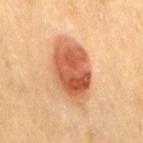workup: total-body-photography surveillance lesion; no biopsy
anatomic site: the right thigh
acquisition: total-body-photography crop, ~15 mm field of view
subject: female, aged approximately 40
automated metrics: an area of roughly 24 mm² and a symmetry-axis asymmetry near 0.1; a lesion–skin lightness drop of about 14 and a lesion-to-skin contrast of about 9.5 (normalized; higher = more distinct); a border-irregularity rating of about 1.5/10, a color-variation rating of about 6/10, and peripheral color asymmetry of about 2
illumination: cross-polarized